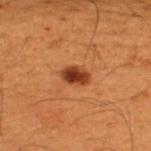The lesion was photographed on a routine skin check and not biopsied; there is no pathology result.
A male subject aged 48–52.
The lesion-visualizer software estimated a footprint of about 5 mm², an outline eccentricity of about 0.75 (0 = round, 1 = elongated), and a symmetry-axis asymmetry near 0.15. And it measured a lesion color around L≈32 a*≈24 b*≈32 in CIELAB, about 13 CIELAB-L* units darker than the surrounding skin, and a lesion-to-skin contrast of about 11.5 (normalized; higher = more distinct).
Cropped from a whole-body photographic skin survey; the tile spans about 15 mm.
From the upper back.
Imaged with cross-polarized lighting.